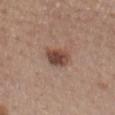Notes:
* notes: imaged on a skin check; not biopsied
* illumination: white-light
* TBP lesion metrics: a footprint of about 7.5 mm², a shape eccentricity near 0.7, and a shape-asymmetry score of about 0.25 (0 = symmetric); a lesion color around L≈46 a*≈20 b*≈26 in CIELAB, about 13 CIELAB-L* units darker than the surrounding skin, and a normalized border contrast of about 9.5; border irregularity of about 2.5 on a 0–10 scale, internal color variation of about 4 on a 0–10 scale, and peripheral color asymmetry of about 1
* subject: female, aged approximately 65
* lesion diameter: ~3.5 mm (longest diameter)
* acquisition: ~15 mm tile from a whole-body skin photo
* site: the back